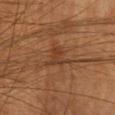Notes:
* notes · total-body-photography surveillance lesion; no biopsy
* subject · female, about 60 years old
* image · ~15 mm tile from a whole-body skin photo
* diameter · ≈3.5 mm
* location · the head or neck
* illumination · cross-polarized